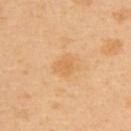notes = imaged on a skin check; not biopsied | lesion diameter = about 2.5 mm | image = total-body-photography crop, ~15 mm field of view | illumination = cross-polarized | body site = the upper back | automated metrics = a footprint of about 4.5 mm², a shape eccentricity near 0.55, and two-axis asymmetry of about 0.35; a color-variation rating of about 1.5/10 and a peripheral color-asymmetry measure near 0.5; an automated nevus-likeness rating near 0 out of 100 and a detector confidence of about 100 out of 100 that the crop contains a lesion | subject = male, in their 40s.